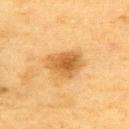biopsy status = imaged on a skin check; not biopsied
image source = 15 mm crop, total-body photography
subject = female, approximately 55 years of age
anatomic site = the upper back
size = ~4.5 mm (longest diameter)
TBP lesion metrics = an average lesion color of about L≈55 a*≈21 b*≈43 (CIELAB), a lesion–skin lightness drop of about 11, and a normalized border contrast of about 8; a nevus-likeness score of about 95/100 and a detector confidence of about 100 out of 100 that the crop contains a lesion